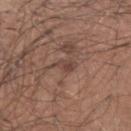Clinical impression: This lesion was catalogued during total-body skin photography and was not selected for biopsy. Acquisition and patient details: On the left upper arm. A region of skin cropped from a whole-body photographic capture, roughly 15 mm wide. The lesion's longest dimension is about 2.5 mm. Automated image analysis of the tile measured a footprint of about 3 mm². The software also gave a lesion color around L≈43 a*≈18 b*≈24 in CIELAB and a lesion–skin lightness drop of about 7. This is a white-light tile. The patient is a male approximately 45 years of age.